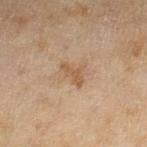notes = total-body-photography surveillance lesion; no biopsy.